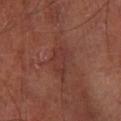Part of a total-body skin-imaging series; this lesion was reviewed on a skin check and was not flagged for biopsy.
The lesion is on the right lower leg.
A male patient in their mid-60s.
This image is a 15 mm lesion crop taken from a total-body photograph.
The tile uses cross-polarized illumination.